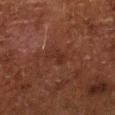Q: Is there a histopathology result?
A: total-body-photography surveillance lesion; no biopsy